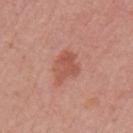Clinical summary: A female patient, in their mid-50s. The lesion is located on the left upper arm. Cropped from a whole-body photographic skin survey; the tile spans about 15 mm.